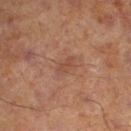<lesion>
<biopsy_status>not biopsied; imaged during a skin examination</biopsy_status>
<patient>
  <sex>male</sex>
  <age_approx>70</age_approx>
</patient>
<site>left lower leg</site>
<image>
  <source>total-body photography crop</source>
  <field_of_view_mm>15</field_of_view_mm>
</image>
</lesion>